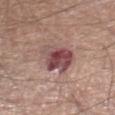<record>
<biopsy_status>not biopsied; imaged during a skin examination</biopsy_status>
<image>
  <source>total-body photography crop</source>
  <field_of_view_mm>15</field_of_view_mm>
</image>
<patient>
  <sex>male</sex>
  <age_approx>65</age_approx>
</patient>
<site>leg</site>
<lesion_size>
  <long_diameter_mm_approx>5.5</long_diameter_mm_approx>
</lesion_size>
</record>